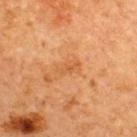Captured during whole-body skin photography for melanoma surveillance; the lesion was not biopsied. This is a cross-polarized tile. From the upper back. A male subject aged 63–67. A roughly 15 mm field-of-view crop from a total-body skin photograph.